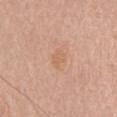This lesion was catalogued during total-body skin photography and was not selected for biopsy.
A region of skin cropped from a whole-body photographic capture, roughly 15 mm wide.
On the chest.
A male patient aged 78 to 82.
Approximately 3 mm at its widest.